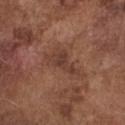  biopsy_status: not biopsied; imaged during a skin examination
  site: front of the torso
  image:
    source: total-body photography crop
    field_of_view_mm: 15
  lighting: white-light
  lesion_size:
    long_diameter_mm_approx: 5.0
  patient:
    sex: male
    age_approx: 75